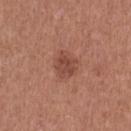Located on the left thigh. A female subject about 50 years old. The recorded lesion diameter is about 3 mm. Cropped from a total-body skin-imaging series; the visible field is about 15 mm. The tile uses white-light illumination.